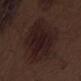Image and clinical context:
Approximately 8.5 mm at its widest. The lesion is located on the right thigh. The tile uses white-light illumination. Cropped from a total-body skin-imaging series; the visible field is about 15 mm. The subject is a male aged 68 to 72.